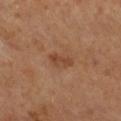follow-up: imaged on a skin check; not biopsied
image: 15 mm crop, total-body photography
subject: female, in their mid- to late 60s
anatomic site: the right lower leg
lighting: cross-polarized